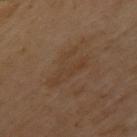Recorded during total-body skin imaging; not selected for excision or biopsy. Located on the upper back. The lesion's longest dimension is about 5.5 mm. The tile uses cross-polarized illumination. Cropped from a total-body skin-imaging series; the visible field is about 15 mm. The subject is a female in their 60s.